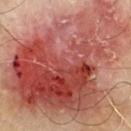| field | value |
|---|---|
| notes | no biopsy performed (imaged during a skin exam) |
| tile lighting | cross-polarized illumination |
| subject | male, aged 68 to 72 |
| size | ≈16.5 mm |
| location | the front of the torso |
| image source | 15 mm crop, total-body photography |